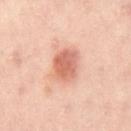Recorded during total-body skin imaging; not selected for excision or biopsy.
The lesion is located on the left leg.
Automated tile analysis of the lesion measured a footprint of about 9.5 mm², an eccentricity of roughly 0.7, and two-axis asymmetry of about 0.15. The software also gave roughly 13 lightness units darker than nearby skin. And it measured border irregularity of about 1.5 on a 0–10 scale, a color-variation rating of about 4.5/10, and a peripheral color-asymmetry measure near 1.5. It also reported a nevus-likeness score of about 95/100.
This is a cross-polarized tile.
This image is a 15 mm lesion crop taken from a total-body photograph.
Measured at roughly 4 mm in maximum diameter.
A female patient, about 40 years old.